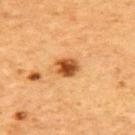subject = female, aged 58 to 62
location = the upper back
image source = ~15 mm crop, total-body skin-cancer survey